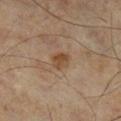  biopsy_status: not biopsied; imaged during a skin examination
  lighting: cross-polarized
  image:
    source: total-body photography crop
    field_of_view_mm: 15
  patient:
    sex: male
    age_approx: 60
  lesion_size:
    long_diameter_mm_approx: 3.0
  site: leg